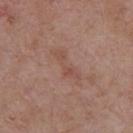Findings:
– biopsy status — catalogued during a skin exam; not biopsied
– location — the upper back
– subject — female, approximately 60 years of age
– lighting — white-light
– automated lesion analysis — a footprint of about 6 mm², a shape eccentricity near 0.95, and a shape-asymmetry score of about 0.5 (0 = symmetric); a nevus-likeness score of about 0/100
– image source — ~15 mm crop, total-body skin-cancer survey
– size — about 5 mm